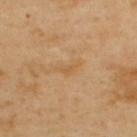No biopsy was performed on this lesion — it was imaged during a full skin examination and was not determined to be concerning.
Cropped from a total-body skin-imaging series; the visible field is about 15 mm.
This is a cross-polarized tile.
The total-body-photography lesion software estimated a footprint of about 3 mm² and two-axis asymmetry of about 0.45. The analysis additionally found an average lesion color of about L≈59 a*≈19 b*≈41 (CIELAB), about 6 CIELAB-L* units darker than the surrounding skin, and a normalized lesion–skin contrast near 5. The analysis additionally found a border-irregularity index near 5/10, a color-variation rating of about 0.5/10, and radial color variation of about 0. And it measured a classifier nevus-likeness of about 0/100 and lesion-presence confidence of about 100/100.
From the upper back.
A male patient, approximately 55 years of age.